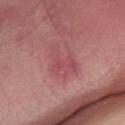Recorded during total-body skin imaging; not selected for excision or biopsy. A 15 mm close-up tile from a total-body photography series done for melanoma screening. A male patient, aged 33–37. This is a white-light tile. Measured at roughly 4.5 mm in maximum diameter. From the right lower leg.